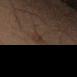notes = total-body-photography surveillance lesion; no biopsy
diameter = about 2.5 mm
tile lighting = cross-polarized
anatomic site = the left thigh
patient = male, roughly 65 years of age
automated metrics = a mean CIELAB color near L≈23 a*≈12 b*≈18, about 4 CIELAB-L* units darker than the surrounding skin, and a normalized lesion–skin contrast near 5.5; a border-irregularity index near 2/10, internal color variation of about 1 on a 0–10 scale, and a peripheral color-asymmetry measure near 0.5; lesion-presence confidence of about 90/100
imaging modality = ~15 mm crop, total-body skin-cancer survey